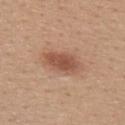Part of a total-body skin-imaging series; this lesion was reviewed on a skin check and was not flagged for biopsy. About 4.5 mm across. Automated tile analysis of the lesion measured an average lesion color of about L≈52 a*≈22 b*≈30 (CIELAB), a lesion–skin lightness drop of about 11, and a lesion-to-skin contrast of about 8 (normalized; higher = more distinct). The analysis additionally found a border-irregularity index near 2/10, a within-lesion color-variation index near 3/10, and peripheral color asymmetry of about 1. Located on the back. A 15 mm close-up extracted from a 3D total-body photography capture. A female subject roughly 30 years of age. Imaged with white-light lighting.